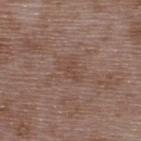Part of a total-body skin-imaging series; this lesion was reviewed on a skin check and was not flagged for biopsy.
A male patient aged 48 to 52.
A 15 mm close-up extracted from a 3D total-body photography capture.
Located on the upper back.